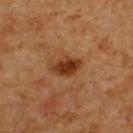Clinical impression: Imaged during a routine full-body skin examination; the lesion was not biopsied and no histopathology is available. Image and clinical context: A male subject, about 60 years old. From the upper back. A lesion tile, about 15 mm wide, cut from a 3D total-body photograph.